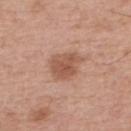image source: ~15 mm crop, total-body skin-cancer survey | tile lighting: white-light | automated lesion analysis: a mean CIELAB color near L≈54 a*≈22 b*≈30, roughly 10 lightness units darker than nearby skin, and a lesion-to-skin contrast of about 7.5 (normalized; higher = more distinct); a nevus-likeness score of about 75/100 and lesion-presence confidence of about 100/100 | body site: the upper back | lesion size: about 4 mm | patient: male, roughly 65 years of age.